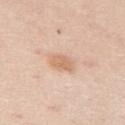Assessment:
Part of a total-body skin-imaging series; this lesion was reviewed on a skin check and was not flagged for biopsy.
Clinical summary:
A close-up tile cropped from a whole-body skin photograph, about 15 mm across. The lesion is on the arm. A male patient, aged 33 to 37.